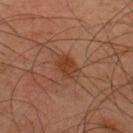Approximately 3 mm at its widest. Cropped from a total-body skin-imaging series; the visible field is about 15 mm. The lesion is located on the upper back. A male subject, approximately 45 years of age.